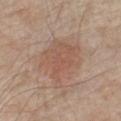Impression: Imaged during a routine full-body skin examination; the lesion was not biopsied and no histopathology is available. Clinical summary: The lesion is on the chest. Imaged with white-light lighting. The recorded lesion diameter is about 5.5 mm. The patient is a male roughly 80 years of age. A 15 mm close-up tile from a total-body photography series done for melanoma screening. Automated image analysis of the tile measured a lesion color around L≈55 a*≈19 b*≈28 in CIELAB, a lesion–skin lightness drop of about 7, and a normalized border contrast of about 5. The software also gave a nevus-likeness score of about 90/100.